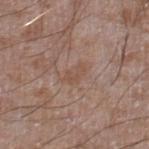Assessment:
The lesion was photographed on a routine skin check and not biopsied; there is no pathology result.
Image and clinical context:
Imaged with white-light lighting. A close-up tile cropped from a whole-body skin photograph, about 15 mm across. The lesion's longest dimension is about 2.5 mm. Located on the right lower leg. A male patient roughly 60 years of age.